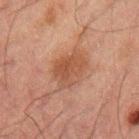Part of a total-body skin-imaging series; this lesion was reviewed on a skin check and was not flagged for biopsy. Cropped from a total-body skin-imaging series; the visible field is about 15 mm. A male patient, aged around 50. The recorded lesion diameter is about 4.5 mm. Captured under cross-polarized illumination. On the left upper arm.